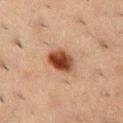Q: Was this lesion biopsied?
A: total-body-photography surveillance lesion; no biopsy
Q: How was this image acquired?
A: 15 mm crop, total-body photography
Q: Lesion location?
A: the chest
Q: How large is the lesion?
A: ≈3.5 mm
Q: Patient demographics?
A: male, in their 50s
Q: How was the tile lit?
A: cross-polarized illumination